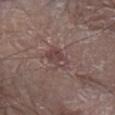biopsy status — imaged on a skin check; not biopsied
site — the arm
patient — male, about 80 years old
imaging modality — total-body-photography crop, ~15 mm field of view
image-analysis metrics — a lesion area of about 5.5 mm², a shape eccentricity near 0.8, and a symmetry-axis asymmetry near 0.3; a border-irregularity rating of about 3/10, a color-variation rating of about 4.5/10, and peripheral color asymmetry of about 1.5
lighting — white-light illumination